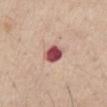Part of a total-body skin-imaging series; this lesion was reviewed on a skin check and was not flagged for biopsy.
Approximately 2.5 mm at its widest.
Imaged with white-light lighting.
Located on the front of the torso.
This image is a 15 mm lesion crop taken from a total-body photograph.
A male subject aged around 55.